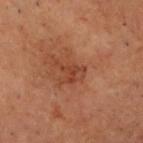Recorded during total-body skin imaging; not selected for excision or biopsy.
The lesion is on the head or neck.
Longest diameter approximately 4 mm.
Automated image analysis of the tile measured an area of roughly 5.5 mm², a shape eccentricity near 0.85, and a shape-asymmetry score of about 0.55 (0 = symmetric). The software also gave roughly 6 lightness units darker than nearby skin and a normalized lesion–skin contrast near 6. And it measured a nevus-likeness score of about 0/100 and a lesion-detection confidence of about 100/100.
A 15 mm close-up tile from a total-body photography series done for melanoma screening.
This is a cross-polarized tile.
A male patient, aged 68–72.